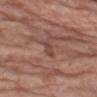The lesion was photographed on a routine skin check and not biopsied; there is no pathology result. A male patient aged 68 to 72. Located on the left upper arm. A 15 mm close-up tile from a total-body photography series done for melanoma screening.